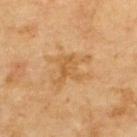Assessment:
Imaged during a routine full-body skin examination; the lesion was not biopsied and no histopathology is available.
Acquisition and patient details:
The total-body-photography lesion software estimated an area of roughly 9 mm². And it measured a lesion color around L≈56 a*≈19 b*≈41 in CIELAB and about 7 CIELAB-L* units darker than the surrounding skin. The software also gave a border-irregularity rating of about 8/10 and radial color variation of about 1. This image is a 15 mm lesion crop taken from a total-body photograph. From the back. A male subject aged 68–72.